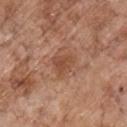This lesion was catalogued during total-body skin photography and was not selected for biopsy. On the chest. Longest diameter approximately 3 mm. This is a white-light tile. The subject is a male in their 70s. A 15 mm close-up tile from a total-body photography series done for melanoma screening.